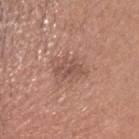<tbp_lesion>
<biopsy_status>not biopsied; imaged during a skin examination</biopsy_status>
<site>head or neck</site>
<image>
  <source>total-body photography crop</source>
  <field_of_view_mm>15</field_of_view_mm>
</image>
<patient>
  <sex>female</sex>
  <age_approx>40</age_approx>
</patient>
<automated_metrics>
  <nevus_likeness_0_100>0</nevus_likeness_0_100>
  <lesion_detection_confidence_0_100>100</lesion_detection_confidence_0_100>
</automated_metrics>
</tbp_lesion>